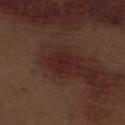Image and clinical context: Imaged with white-light lighting. Cropped from a total-body skin-imaging series; the visible field is about 15 mm. The lesion is located on the left thigh. A male patient, approximately 70 years of age.